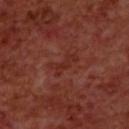workup = catalogued during a skin exam; not biopsied
subject = male, roughly 70 years of age
anatomic site = the upper back
lesion size = ~3.5 mm (longest diameter)
lighting = cross-polarized
image source = ~15 mm crop, total-body skin-cancer survey